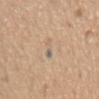Q: Was a biopsy performed?
A: total-body-photography surveillance lesion; no biopsy
Q: Who is the patient?
A: male, in their 70s
Q: Where on the body is the lesion?
A: the front of the torso
Q: Automated lesion metrics?
A: a footprint of about 3 mm², an outline eccentricity of about 0.95 (0 = round, 1 = elongated), and a symmetry-axis asymmetry near 0.4; a nevus-likeness score of about 0/100 and a detector confidence of about 55 out of 100 that the crop contains a lesion
Q: What lighting was used for the tile?
A: white-light
Q: Lesion size?
A: about 3.5 mm
Q: How was this image acquired?
A: ~15 mm crop, total-body skin-cancer survey